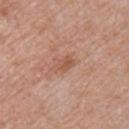Q: Was this lesion biopsied?
A: catalogued during a skin exam; not biopsied
Q: What did automated image analysis measure?
A: a mean CIELAB color near L≈54 a*≈23 b*≈30 and a normalized lesion–skin contrast near 6
Q: How large is the lesion?
A: ≈3 mm
Q: How was this image acquired?
A: ~15 mm crop, total-body skin-cancer survey
Q: Patient demographics?
A: male, about 50 years old
Q: Illumination type?
A: white-light
Q: Where on the body is the lesion?
A: the right upper arm